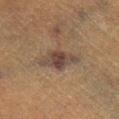Captured during whole-body skin photography for melanoma surveillance; the lesion was not biopsied. Longest diameter approximately 5 mm. A male patient, aged 48–52. On the right lower leg. This image is a 15 mm lesion crop taken from a total-body photograph.